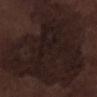  site: left lower leg
  automated_metrics:
    area_mm2_approx: 130.0
    eccentricity: 0.55
    shape_asymmetry: 0.5
    cielab_L: 18
    cielab_a: 13
    cielab_b: 14
    vs_skin_contrast_norm: 9.0
    nevus_likeness_0_100: 5
  image:
    source: total-body photography crop
    field_of_view_mm: 15
  lighting: white-light
  patient:
    sex: male
    age_approx: 70
  lesion_size:
    long_diameter_mm_approx: 18.0